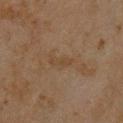Assessment: Imaged during a routine full-body skin examination; the lesion was not biopsied and no histopathology is available. Clinical summary: A roughly 15 mm field-of-view crop from a total-body skin photograph. The subject is a male approximately 45 years of age. From the chest. Imaged with cross-polarized lighting. Approximately 3.5 mm at its widest.